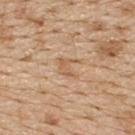Impression:
Imaged during a routine full-body skin examination; the lesion was not biopsied and no histopathology is available.
Acquisition and patient details:
An algorithmic analysis of the crop reported a footprint of about 4.5 mm², an eccentricity of roughly 0.5, and a shape-asymmetry score of about 0.4 (0 = symmetric). The software also gave a normalized border contrast of about 5.5. It also reported border irregularity of about 5.5 on a 0–10 scale, internal color variation of about 2 on a 0–10 scale, and radial color variation of about 0.5. The analysis additionally found a nevus-likeness score of about 0/100 and a detector confidence of about 95 out of 100 that the crop contains a lesion. The recorded lesion diameter is about 2.5 mm. A 15 mm crop from a total-body photograph taken for skin-cancer surveillance. Captured under white-light illumination. The patient is a male approximately 70 years of age. The lesion is on the upper back.